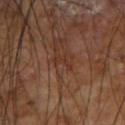Context: From the right forearm. A roughly 15 mm field-of-view crop from a total-body skin photograph. A male subject in their mid-60s. About 2.5 mm across. The lesion-visualizer software estimated a mean CIELAB color near L≈34 a*≈22 b*≈27, a lesion–skin lightness drop of about 6, and a normalized border contrast of about 5.5. And it measured border irregularity of about 6 on a 0–10 scale, a color-variation rating of about 0/10, and peripheral color asymmetry of about 0.